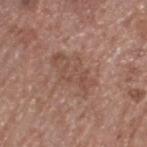biopsy status: no biopsy performed (imaged during a skin exam) | image-analysis metrics: an average lesion color of about L≈50 a*≈19 b*≈26 (CIELAB) and a normalized lesion–skin contrast near 5; border irregularity of about 5 on a 0–10 scale and radial color variation of about 1; a classifier nevus-likeness of about 0/100 | lesion diameter: ≈6 mm | site: the head or neck | patient: male, roughly 60 years of age | illumination: white-light | imaging modality: 15 mm crop, total-body photography.